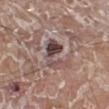workup: catalogued during a skin exam; not biopsied | patient: male, aged 78 to 82 | anatomic site: the left lower leg | lighting: white-light | image: ~15 mm crop, total-body skin-cancer survey | size: ≈4 mm.